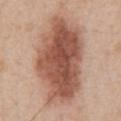Imaged during a routine full-body skin examination; the lesion was not biopsied and no histopathology is available.
The lesion is on the chest.
Automated image analysis of the tile measured an area of roughly 50 mm², an eccentricity of roughly 0.85, and a shape-asymmetry score of about 0.2 (0 = symmetric). And it measured a mean CIELAB color near L≈54 a*≈22 b*≈29 and a lesion–skin lightness drop of about 16. The analysis additionally found border irregularity of about 2.5 on a 0–10 scale, internal color variation of about 6.5 on a 0–10 scale, and a peripheral color-asymmetry measure near 2.5.
A male subject in their mid- to late 50s.
Imaged with white-light lighting.
Longest diameter approximately 11.5 mm.
A 15 mm close-up tile from a total-body photography series done for melanoma screening.